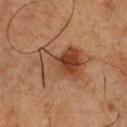Recorded during total-body skin imaging; not selected for excision or biopsy. Measured at roughly 7 mm in maximum diameter. The total-body-photography lesion software estimated an automated nevus-likeness rating near 95 out of 100 and a detector confidence of about 100 out of 100 that the crop contains a lesion. Captured under cross-polarized illumination. The patient is a male approximately 50 years of age. Located on the chest. This image is a 15 mm lesion crop taken from a total-body photograph.